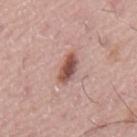<record>
  <biopsy_status>not biopsied; imaged during a skin examination</biopsy_status>
  <site>back</site>
  <automated_metrics>
    <cielab_L>52</cielab_L>
    <cielab_a>23</cielab_a>
    <cielab_b>25</cielab_b>
    <vs_skin_darker_L>15.0</vs_skin_darker_L>
    <vs_skin_contrast_norm>10.0</vs_skin_contrast_norm>
    <color_variation_0_10>5.0</color_variation_0_10>
    <peripheral_color_asymmetry>1.5</peripheral_color_asymmetry>
  </automated_metrics>
  <lesion_size>
    <long_diameter_mm_approx>3.5</long_diameter_mm_approx>
  </lesion_size>
  <patient>
    <sex>male</sex>
    <age_approx>75</age_approx>
  </patient>
  <image>
    <source>total-body photography crop</source>
    <field_of_view_mm>15</field_of_view_mm>
  </image>
  <lighting>white-light</lighting>
</record>